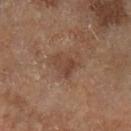{"lighting": "cross-polarized", "patient": {"sex": "male", "age_approx": 70}, "lesion_size": {"long_diameter_mm_approx": 3.0}, "image": {"source": "total-body photography crop", "field_of_view_mm": 15}, "site": "left lower leg"}